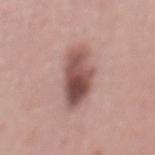Assessment: No biopsy was performed on this lesion — it was imaged during a full skin examination and was not determined to be concerning. Background: Located on the mid back. Longest diameter approximately 6.5 mm. The lesion-visualizer software estimated an average lesion color of about L≈51 a*≈21 b*≈22 (CIELAB) and a normalized border contrast of about 10. It also reported a classifier nevus-likeness of about 85/100. A male subject in their 40s. A roughly 15 mm field-of-view crop from a total-body skin photograph.